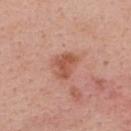Findings:
* notes · total-body-photography surveillance lesion; no biopsy
* body site · the upper back
* subject · female, aged 33 to 37
* size · ~3.5 mm (longest diameter)
* image source · ~15 mm tile from a whole-body skin photo
* tile lighting · white-light